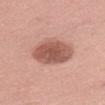Clinical impression:
The lesion was tiled from a total-body skin photograph and was not biopsied.
Clinical summary:
Located on the left upper arm. Captured under white-light illumination. Measured at roughly 5.5 mm in maximum diameter. A 15 mm close-up extracted from a 3D total-body photography capture. A female patient, aged 48 to 52.